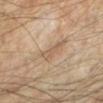Notes:
* follow-up · imaged on a skin check; not biopsied